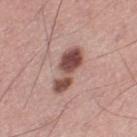The lesion was tiled from a total-body skin photograph and was not biopsied.
Measured at roughly 5.5 mm in maximum diameter.
A male patient, in their 60s.
The lesion is located on the left thigh.
An algorithmic analysis of the crop reported a lesion area of about 11 mm², an outline eccentricity of about 0.9 (0 = round, 1 = elongated), and a symmetry-axis asymmetry near 0.35. The software also gave border irregularity of about 4.5 on a 0–10 scale, a color-variation rating of about 5/10, and radial color variation of about 1.5. The software also gave an automated nevus-likeness rating near 80 out of 100.
Cropped from a total-body skin-imaging series; the visible field is about 15 mm.
This is a white-light tile.